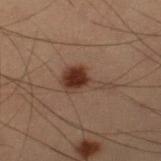Notes:
- biopsy status · no biopsy performed (imaged during a skin exam)
- imaging modality · ~15 mm tile from a whole-body skin photo
- illumination · cross-polarized illumination
- subject · male, aged around 55
- automated lesion analysis · a footprint of about 8.5 mm² and a shape eccentricity near 0.9; internal color variation of about 3.5 on a 0–10 scale and radial color variation of about 1.5; a nevus-likeness score of about 100/100
- location · the right lower leg